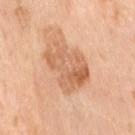automated metrics=a normalized border contrast of about 6.5; a peripheral color-asymmetry measure near 2; a classifier nevus-likeness of about 0/100 and a lesion-detection confidence of about 100/100 | acquisition=~15 mm crop, total-body skin-cancer survey | illumination=cross-polarized | patient=female, aged approximately 55 | anatomic site=the right thigh | lesion size=about 6.5 mm.